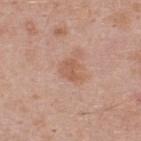<case>
  <biopsy_status>not biopsied; imaged during a skin examination</biopsy_status>
  <image>
    <source>total-body photography crop</source>
    <field_of_view_mm>15</field_of_view_mm>
  </image>
  <patient>
    <sex>male</sex>
    <age_approx>45</age_approx>
  </patient>
  <site>upper back</site>
  <lighting>white-light</lighting>
</case>